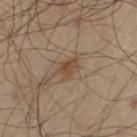Impression:
The lesion was photographed on a routine skin check and not biopsied; there is no pathology result.
Context:
Longest diameter approximately 3 mm. The lesion is located on the right thigh. Imaged with cross-polarized lighting. A male patient about 55 years old. A lesion tile, about 15 mm wide, cut from a 3D total-body photograph.